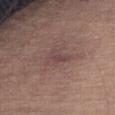follow-up: imaged on a skin check; not biopsied | diameter: about 3.5 mm | TBP lesion metrics: an area of roughly 5.5 mm², an outline eccentricity of about 0.8 (0 = round, 1 = elongated), and two-axis asymmetry of about 0.4; border irregularity of about 4.5 on a 0–10 scale, a color-variation rating of about 2/10, and radial color variation of about 0.5 | site: the leg | illumination: white-light illumination | imaging modality: ~15 mm crop, total-body skin-cancer survey | subject: male, aged around 65.